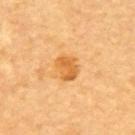Part of a total-body skin-imaging series; this lesion was reviewed on a skin check and was not flagged for biopsy.
The subject is aged around 60.
From the upper back.
Cropped from a total-body skin-imaging series; the visible field is about 15 mm.